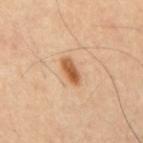Q: What is the anatomic site?
A: the mid back
Q: What lighting was used for the tile?
A: cross-polarized
Q: What did automated image analysis measure?
A: an area of roughly 5 mm², an outline eccentricity of about 0.9 (0 = round, 1 = elongated), and a symmetry-axis asymmetry near 0.25; a color-variation rating of about 4.5/10 and a peripheral color-asymmetry measure near 1.5; an automated nevus-likeness rating near 100 out of 100 and lesion-presence confidence of about 100/100
Q: What kind of image is this?
A: ~15 mm tile from a whole-body skin photo
Q: Patient demographics?
A: male, aged 58–62
Q: How large is the lesion?
A: ≈3.5 mm